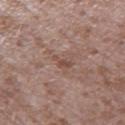Q: Was this lesion biopsied?
A: catalogued during a skin exam; not biopsied
Q: Patient demographics?
A: male, approximately 45 years of age
Q: Lesion location?
A: the left thigh
Q: What did automated image analysis measure?
A: an average lesion color of about L≈48 a*≈19 b*≈25 (CIELAB), about 8 CIELAB-L* units darker than the surrounding skin, and a normalized lesion–skin contrast near 6; a within-lesion color-variation index near 0/10 and radial color variation of about 0
Q: What is the lesion's diameter?
A: about 3 mm
Q: How was the tile lit?
A: white-light
Q: What kind of image is this?
A: ~15 mm tile from a whole-body skin photo